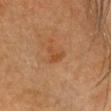Case summary:
– workup — imaged on a skin check; not biopsied
– acquisition — ~15 mm tile from a whole-body skin photo
– automated metrics — an area of roughly 2.5 mm² and a symmetry-axis asymmetry near 0.65; border irregularity of about 7 on a 0–10 scale and internal color variation of about 0.5 on a 0–10 scale
– tile lighting — cross-polarized
– site — the head or neck
– patient — female, roughly 65 years of age
– lesion size — ~3 mm (longest diameter)